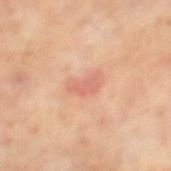* biopsy status · catalogued during a skin exam; not biopsied
* image · ~15 mm tile from a whole-body skin photo
* subject · male, in their 70s
* location · the back